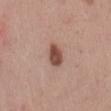Findings:
- lesion size · about 3 mm
- image · 15 mm crop, total-body photography
- anatomic site · the mid back
- automated lesion analysis · border irregularity of about 2 on a 0–10 scale and a peripheral color-asymmetry measure near 1
- subject · male, approximately 50 years of age